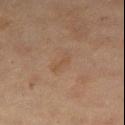This lesion was catalogued during total-body skin photography and was not selected for biopsy. The subject is a female aged 68 to 72. A region of skin cropped from a whole-body photographic capture, roughly 15 mm wide. On the right thigh. Longest diameter approximately 2.5 mm. This is a cross-polarized tile.